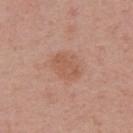Findings:
* biopsy status · total-body-photography surveillance lesion; no biopsy
* automated metrics · a lesion area of about 10 mm², a shape eccentricity near 0.6, and a shape-asymmetry score of about 0.15 (0 = symmetric); a lesion–skin lightness drop of about 6 and a normalized border contrast of about 5.5; a nevus-likeness score of about 5/100 and a detector confidence of about 100 out of 100 that the crop contains a lesion
* imaging modality · total-body-photography crop, ~15 mm field of view
* patient · female, in their mid- to late 50s
* tile lighting · white-light illumination
* lesion size · ~4 mm (longest diameter)
* site · the left upper arm